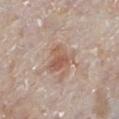Part of a total-body skin-imaging series; this lesion was reviewed on a skin check and was not flagged for biopsy.
Imaged with white-light lighting.
The subject is a male about 80 years old.
A lesion tile, about 15 mm wide, cut from a 3D total-body photograph.
The lesion is located on the left lower leg.
Automated tile analysis of the lesion measured a mean CIELAB color near L≈57 a*≈19 b*≈28, a lesion–skin lightness drop of about 9, and a normalized lesion–skin contrast near 7. The software also gave border irregularity of about 4.5 on a 0–10 scale, a within-lesion color-variation index near 4/10, and radial color variation of about 1.5. The analysis additionally found an automated nevus-likeness rating near 60 out of 100 and a detector confidence of about 100 out of 100 that the crop contains a lesion.